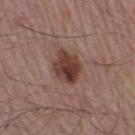Q: Was this lesion biopsied?
A: catalogued during a skin exam; not biopsied
Q: How large is the lesion?
A: ~4.5 mm (longest diameter)
Q: What is the anatomic site?
A: the right thigh
Q: What is the imaging modality?
A: ~15 mm tile from a whole-body skin photo
Q: Patient demographics?
A: male, aged 53–57
Q: How was the tile lit?
A: white-light